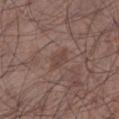Q: Is there a histopathology result?
A: total-body-photography surveillance lesion; no biopsy
Q: Where on the body is the lesion?
A: the left lower leg
Q: What kind of image is this?
A: ~15 mm crop, total-body skin-cancer survey
Q: Who is the patient?
A: male, aged 63 to 67